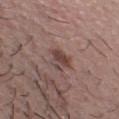The lesion was photographed on a routine skin check and not biopsied; there is no pathology result. A roughly 15 mm field-of-view crop from a total-body skin photograph. The subject is a male aged around 30. Captured under white-light illumination.